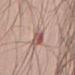notes: imaged on a skin check; not biopsied
image-analysis metrics: an area of roughly 9.5 mm², an outline eccentricity of about 0.85 (0 = round, 1 = elongated), and two-axis asymmetry of about 0.35
imaging modality: total-body-photography crop, ~15 mm field of view
site: the right upper arm
subject: male, aged around 50
lighting: white-light illumination